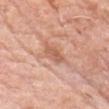lesion_size:
  long_diameter_mm_approx: 3.0
patient:
  sex: male
  age_approx: 75
lighting: white-light
image:
  source: total-body photography crop
  field_of_view_mm: 15
site: head or neck
automated_metrics:
  area_mm2_approx: 4.0
  eccentricity: 0.9
  shape_asymmetry: 0.35